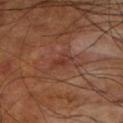This lesion was catalogued during total-body skin photography and was not selected for biopsy.
A male subject, aged 58 to 62.
From the left lower leg.
Cropped from a whole-body photographic skin survey; the tile spans about 15 mm.
The recorded lesion diameter is about 2.5 mm.
Automated tile analysis of the lesion measured an eccentricity of roughly 0.85 and a shape-asymmetry score of about 0.35 (0 = symmetric). The analysis additionally found a lesion color around L≈35 a*≈23 b*≈27 in CIELAB, a lesion–skin lightness drop of about 6, and a normalized lesion–skin contrast near 6. And it measured a border-irregularity rating of about 4/10.